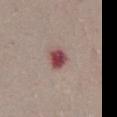{
  "biopsy_status": "not biopsied; imaged during a skin examination",
  "lesion_size": {
    "long_diameter_mm_approx": 2.5
  },
  "site": "abdomen",
  "lighting": "white-light",
  "patient": {
    "sex": "female",
    "age_approx": 50
  },
  "automated_metrics": {
    "vs_skin_darker_L": 16.0,
    "vs_skin_contrast_norm": 11.5,
    "nevus_likeness_0_100": 0
  },
  "image": {
    "source": "total-body photography crop",
    "field_of_view_mm": 15
  }
}